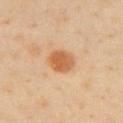Assessment: Recorded during total-body skin imaging; not selected for excision or biopsy. Background: A roughly 15 mm field-of-view crop from a total-body skin photograph. This is a cross-polarized tile. The lesion is located on the front of the torso. The recorded lesion diameter is about 3.5 mm. The subject is a female aged around 50.